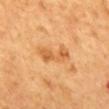biopsy status — no biopsy performed (imaged during a skin exam)
automated metrics — an area of roughly 5.5 mm², a shape eccentricity near 0.95, and a shape-asymmetry score of about 0.4 (0 = symmetric); a mean CIELAB color near L≈61 a*≈26 b*≈46, roughly 10 lightness units darker than nearby skin, and a normalized lesion–skin contrast near 6.5; border irregularity of about 6 on a 0–10 scale, internal color variation of about 2.5 on a 0–10 scale, and radial color variation of about 1
lesion diameter — ~4.5 mm (longest diameter)
body site — the mid back
acquisition — ~15 mm crop, total-body skin-cancer survey
patient — male, aged approximately 65
illumination — cross-polarized illumination